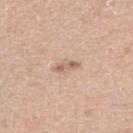Q: Is there a histopathology result?
A: catalogued during a skin exam; not biopsied
Q: What is the imaging modality?
A: 15 mm crop, total-body photography
Q: Who is the patient?
A: female, aged around 70
Q: Automated lesion metrics?
A: an outline eccentricity of about 0.9 (0 = round, 1 = elongated) and two-axis asymmetry of about 0.4; a lesion–skin lightness drop of about 10 and a normalized border contrast of about 6.5
Q: Lesion location?
A: the leg
Q: What is the lesion's diameter?
A: ≈3 mm
Q: Illumination type?
A: white-light